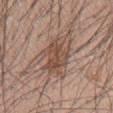Assessment:
No biopsy was performed on this lesion — it was imaged during a full skin examination and was not determined to be concerning.
Background:
Measured at roughly 6 mm in maximum diameter. The patient is a male aged approximately 60. On the mid back. A roughly 15 mm field-of-view crop from a total-body skin photograph. Captured under white-light illumination. Automated image analysis of the tile measured a footprint of about 15 mm², a shape eccentricity near 0.85, and two-axis asymmetry of about 0.3. It also reported a lesion–skin lightness drop of about 10 and a normalized border contrast of about 7.5. The analysis additionally found a color-variation rating of about 4/10 and peripheral color asymmetry of about 1.5. The software also gave a classifier nevus-likeness of about 85/100 and lesion-presence confidence of about 95/100.